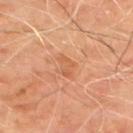Approximately 3 mm at its widest.
A male subject aged around 65.
The lesion is on the upper back.
A 15 mm close-up extracted from a 3D total-body photography capture.
The lesion-visualizer software estimated a mean CIELAB color near L≈57 a*≈25 b*≈37, roughly 6 lightness units darker than nearby skin, and a normalized lesion–skin contrast near 5. It also reported border irregularity of about 3 on a 0–10 scale, a color-variation rating of about 2.5/10, and peripheral color asymmetry of about 1.